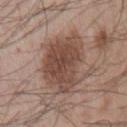Recorded during total-body skin imaging; not selected for excision or biopsy.
This is a white-light tile.
Approximately 7 mm at its widest.
An algorithmic analysis of the crop reported a lesion area of about 28 mm², an outline eccentricity of about 0.6 (0 = round, 1 = elongated), and a symmetry-axis asymmetry near 0.2. It also reported a mean CIELAB color near L≈47 a*≈18 b*≈25, a lesion–skin lightness drop of about 12, and a lesion-to-skin contrast of about 8.5 (normalized; higher = more distinct). It also reported border irregularity of about 3 on a 0–10 scale and radial color variation of about 1.5. The analysis additionally found an automated nevus-likeness rating near 95 out of 100.
A close-up tile cropped from a whole-body skin photograph, about 15 mm across.
On the chest.
The subject is a male aged approximately 55.